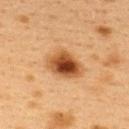biopsy status: imaged on a skin check; not biopsied
subject: female, aged around 40
automated lesion analysis: an area of roughly 12 mm², an eccentricity of roughly 0.7, and two-axis asymmetry of about 0.2; a lesion–skin lightness drop of about 14 and a lesion-to-skin contrast of about 10.5 (normalized; higher = more distinct); a nevus-likeness score of about 100/100 and a lesion-detection confidence of about 100/100
site: the upper back
image source: 15 mm crop, total-body photography
illumination: cross-polarized illumination